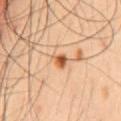Captured during whole-body skin photography for melanoma surveillance; the lesion was not biopsied.
The patient is a male aged 33 to 37.
From the chest.
The total-body-photography lesion software estimated a border-irregularity rating of about 2.5/10, a color-variation rating of about 4.5/10, and a peripheral color-asymmetry measure near 1.5. And it measured a nevus-likeness score of about 100/100 and a lesion-detection confidence of about 100/100.
The recorded lesion diameter is about 2 mm.
This image is a 15 mm lesion crop taken from a total-body photograph.
This is a cross-polarized tile.